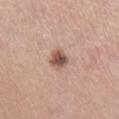Findings:
• follow-up — catalogued during a skin exam; not biopsied
• anatomic site — the leg
• diameter — ≈2.5 mm
• lighting — white-light
• image — total-body-photography crop, ~15 mm field of view
• subject — female, aged 53–57